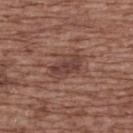{"biopsy_status": "not biopsied; imaged during a skin examination", "patient": {"sex": "female", "age_approx": 75}, "image": {"source": "total-body photography crop", "field_of_view_mm": 15}, "lesion_size": {"long_diameter_mm_approx": 4.5}, "automated_metrics": {"area_mm2_approx": 8.0, "shape_asymmetry": 0.2, "lesion_detection_confidence_0_100": 95}, "site": "upper back", "lighting": "white-light"}